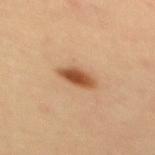biopsy_status: not biopsied; imaged during a skin examination
image:
  source: total-body photography crop
  field_of_view_mm: 15
patient:
  sex: female
  age_approx: 35
site: upper back